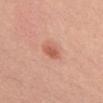| field | value |
|---|---|
| illumination | white-light |
| site | the front of the torso |
| lesion diameter | ~3 mm (longest diameter) |
| image | 15 mm crop, total-body photography |
| patient | male, approximately 30 years of age |